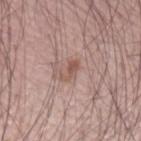workup: no biopsy performed (imaged during a skin exam)
acquisition: ~15 mm crop, total-body skin-cancer survey
body site: the arm
subject: male, in their 70s
diameter: ≈2.5 mm